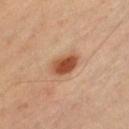Clinical impression:
The lesion was photographed on a routine skin check and not biopsied; there is no pathology result.
Context:
Automated image analysis of the tile measured an average lesion color of about L≈49 a*≈24 b*≈34 (CIELAB) and about 14 CIELAB-L* units darker than the surrounding skin. The software also gave a nevus-likeness score of about 100/100 and a lesion-detection confidence of about 100/100. A male patient aged 33–37. Longest diameter approximately 4 mm. The lesion is on the chest. A roughly 15 mm field-of-view crop from a total-body skin photograph. The tile uses cross-polarized illumination.